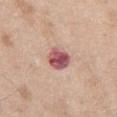Captured during whole-body skin photography for melanoma surveillance; the lesion was not biopsied. Cropped from a whole-body photographic skin survey; the tile spans about 15 mm. Located on the chest. The tile uses white-light illumination. The lesion's longest dimension is about 3.5 mm. The total-body-photography lesion software estimated a lesion area of about 7 mm², an eccentricity of roughly 0.7, and a shape-asymmetry score of about 0.2 (0 = symmetric). It also reported a mean CIELAB color near L≈54 a*≈28 b*≈20. A male subject, in their mid- to late 60s.